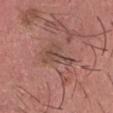Q: Was a biopsy performed?
A: catalogued during a skin exam; not biopsied
Q: What is the imaging modality?
A: 15 mm crop, total-body photography
Q: Patient demographics?
A: male, aged approximately 30
Q: Where on the body is the lesion?
A: the head or neck
Q: How large is the lesion?
A: ~5 mm (longest diameter)